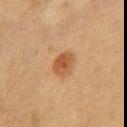Q: Was this lesion biopsied?
A: imaged on a skin check; not biopsied
Q: How was this image acquired?
A: ~15 mm crop, total-body skin-cancer survey
Q: Where on the body is the lesion?
A: the chest
Q: Patient demographics?
A: female, roughly 40 years of age
Q: What is the lesion's diameter?
A: ~2.5 mm (longest diameter)